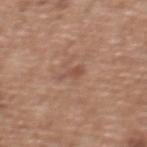Recorded during total-body skin imaging; not selected for excision or biopsy.
Approximately 2.5 mm at its widest.
On the upper back.
A female subject roughly 60 years of age.
A lesion tile, about 15 mm wide, cut from a 3D total-body photograph.
Imaged with white-light lighting.